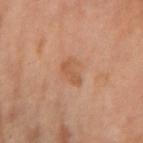The subject is a female about 65 years old.
The lesion's longest dimension is about 3.5 mm.
A 15 mm crop from a total-body photograph taken for skin-cancer surveillance.
The lesion is located on the left forearm.
Imaged with cross-polarized lighting.